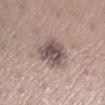A close-up tile cropped from a whole-body skin photograph, about 15 mm across. An algorithmic analysis of the crop reported a lesion area of about 11 mm², a shape eccentricity near 0.5, and two-axis asymmetry of about 0.2. And it measured a border-irregularity index near 2.5/10 and peripheral color asymmetry of about 1.5. A female subject, aged around 45. This is a white-light tile. On the left lower leg.